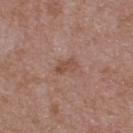Impression:
Captured during whole-body skin photography for melanoma surveillance; the lesion was not biopsied.
Context:
A 15 mm close-up extracted from a 3D total-body photography capture. The recorded lesion diameter is about 3 mm. Located on the upper back. A male subject roughly 50 years of age.